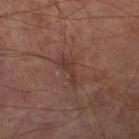The subject is a male approximately 70 years of age. The tile uses cross-polarized illumination. Automated tile analysis of the lesion measured an average lesion color of about L≈35 a*≈21 b*≈22 (CIELAB) and a normalized lesion–skin contrast near 6. The lesion is located on the right lower leg. A 15 mm close-up extracted from a 3D total-body photography capture. Approximately 3.5 mm at its widest.